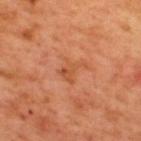<case>
<biopsy_status>not biopsied; imaged during a skin examination</biopsy_status>
<image>
  <source>total-body photography crop</source>
  <field_of_view_mm>15</field_of_view_mm>
</image>
<site>upper back</site>
<patient>
  <sex>male</sex>
  <age_approx>50</age_approx>
</patient>
</case>